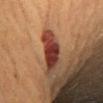Imaged during a routine full-body skin examination; the lesion was not biopsied and no histopathology is available. A 15 mm close-up tile from a total-body photography series done for melanoma screening. The patient is a female aged 58 to 62. The lesion is located on the front of the torso.